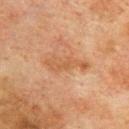notes: total-body-photography surveillance lesion; no biopsy
site: the back
image source: ~15 mm crop, total-body skin-cancer survey
subject: male, aged approximately 75